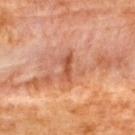Q: Was a biopsy performed?
A: catalogued during a skin exam; not biopsied
Q: What is the anatomic site?
A: the back
Q: How was the tile lit?
A: cross-polarized
Q: What kind of image is this?
A: total-body-photography crop, ~15 mm field of view
Q: What are the patient's age and sex?
A: female, about 65 years old